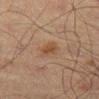Clinical impression:
Recorded during total-body skin imaging; not selected for excision or biopsy.
Clinical summary:
The lesion's longest dimension is about 3 mm. On the left thigh. Cropped from a total-body skin-imaging series; the visible field is about 15 mm. The patient is a male aged approximately 70. The tile uses cross-polarized illumination.